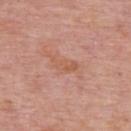| key | value |
|---|---|
| biopsy status | catalogued during a skin exam; not biopsied |
| image | ~15 mm tile from a whole-body skin photo |
| illumination | white-light illumination |
| subject | male, aged approximately 75 |
| lesion size | ~3.5 mm (longest diameter) |
| site | the upper back |